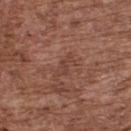{"biopsy_status": "not biopsied; imaged during a skin examination", "lighting": "white-light", "image": {"source": "total-body photography crop", "field_of_view_mm": 15}, "patient": {"sex": "male", "age_approx": 75}, "site": "upper back", "lesion_size": {"long_diameter_mm_approx": 2.5}}